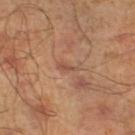Imaged during a routine full-body skin examination; the lesion was not biopsied and no histopathology is available.
Located on the left lower leg.
The lesion-visualizer software estimated a mean CIELAB color near L≈51 a*≈21 b*≈30, roughly 6 lightness units darker than nearby skin, and a normalized border contrast of about 4.5.
About 2.5 mm across.
A male subject, aged 43–47.
The tile uses cross-polarized illumination.
Cropped from a total-body skin-imaging series; the visible field is about 15 mm.